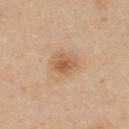{"biopsy_status": "not biopsied; imaged during a skin examination", "lighting": "white-light", "image": {"source": "total-body photography crop", "field_of_view_mm": 15}, "site": "upper back", "automated_metrics": {"cielab_L": 59, "cielab_a": 20, "cielab_b": 36, "vs_skin_darker_L": 10.0, "vs_skin_contrast_norm": 6.5, "lesion_detection_confidence_0_100": 100}, "patient": {"sex": "female", "age_approx": 25}}